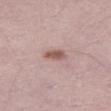Impression: This lesion was catalogued during total-body skin photography and was not selected for biopsy. Background: A male patient approximately 30 years of age. Automated tile analysis of the lesion measured border irregularity of about 2 on a 0–10 scale. And it measured a nevus-likeness score of about 60/100. A region of skin cropped from a whole-body photographic capture, roughly 15 mm wide. Imaged with white-light lighting.